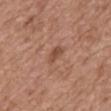The lesion was tiled from a total-body skin photograph and was not biopsied. On the mid back. The subject is a female in their mid- to late 70s. An algorithmic analysis of the crop reported border irregularity of about 4.5 on a 0–10 scale. The software also gave a classifier nevus-likeness of about 5/100 and a lesion-detection confidence of about 100/100. A 15 mm crop from a total-body photograph taken for skin-cancer surveillance.